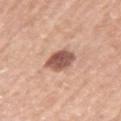Assessment: This lesion was catalogued during total-body skin photography and was not selected for biopsy. Clinical summary: A lesion tile, about 15 mm wide, cut from a 3D total-body photograph. The lesion is located on the left upper arm. The lesion's longest dimension is about 3.5 mm. A male patient aged 63 to 67. This is a white-light tile.